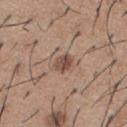Assessment: Part of a total-body skin-imaging series; this lesion was reviewed on a skin check and was not flagged for biopsy. Acquisition and patient details: Captured under white-light illumination. On the front of the torso. The recorded lesion diameter is about 2.5 mm. Cropped from a total-body skin-imaging series; the visible field is about 15 mm. A male patient approximately 60 years of age.